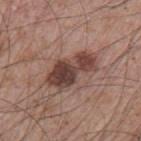Acquisition and patient details: A lesion tile, about 15 mm wide, cut from a 3D total-body photograph. Automated image analysis of the tile measured a lesion color around L≈42 a*≈20 b*≈23 in CIELAB, roughly 14 lightness units darker than nearby skin, and a normalized border contrast of about 10.5. A male patient, aged around 65. The lesion is on the left upper arm. Imaged with white-light lighting.